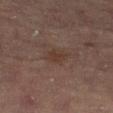Captured during whole-body skin photography for melanoma surveillance; the lesion was not biopsied. Cropped from a whole-body photographic skin survey; the tile spans about 15 mm. Located on the left lower leg. A female subject approximately 70 years of age.